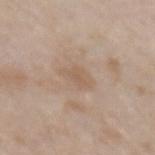Longest diameter approximately 3 mm.
A lesion tile, about 15 mm wide, cut from a 3D total-body photograph.
Captured under white-light illumination.
The patient is a male aged around 45.
On the left forearm.